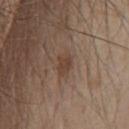Imaged during a routine full-body skin examination; the lesion was not biopsied and no histopathology is available.
A close-up tile cropped from a whole-body skin photograph, about 15 mm across.
On the chest.
An algorithmic analysis of the crop reported a mean CIELAB color near L≈41 a*≈17 b*≈26, roughly 8 lightness units darker than nearby skin, and a normalized border contrast of about 6.5. It also reported border irregularity of about 2.5 on a 0–10 scale, internal color variation of about 1.5 on a 0–10 scale, and radial color variation of about 0.5. The analysis additionally found a nevus-likeness score of about 45/100 and a detector confidence of about 100 out of 100 that the crop contains a lesion.
Imaged with white-light lighting.
A male subject roughly 80 years of age.